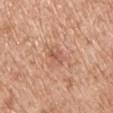| key | value |
|---|---|
| notes | imaged on a skin check; not biopsied |
| lesion size | about 3 mm |
| imaging modality | ~15 mm tile from a whole-body skin photo |
| subject | male, in their mid-70s |
| illumination | white-light illumination |
| anatomic site | the chest |
| automated lesion analysis | a lesion area of about 2.5 mm², an eccentricity of roughly 0.9, and two-axis asymmetry of about 0.35; a color-variation rating of about 0.5/10 and radial color variation of about 0; a nevus-likeness score of about 0/100 and a detector confidence of about 100 out of 100 that the crop contains a lesion |